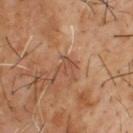No biopsy was performed on this lesion — it was imaged during a full skin examination and was not determined to be concerning. The tile uses cross-polarized illumination. Located on the upper back. The patient is a male about 65 years old. A 15 mm close-up extracted from a 3D total-body photography capture. The recorded lesion diameter is about 3 mm.